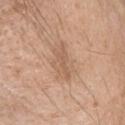Part of a total-body skin-imaging series; this lesion was reviewed on a skin check and was not flagged for biopsy.
This image is a 15 mm lesion crop taken from a total-body photograph.
The subject is a female aged approximately 70.
Imaged with white-light lighting.
The lesion is on the head or neck.
Automated image analysis of the tile measured an automated nevus-likeness rating near 0 out of 100 and a detector confidence of about 100 out of 100 that the crop contains a lesion.